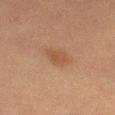Findings:
– follow-up · imaged on a skin check; not biopsied